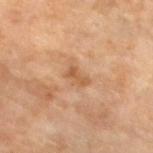Clinical impression:
This lesion was catalogued during total-body skin photography and was not selected for biopsy.
Image and clinical context:
On the left lower leg. A roughly 15 mm field-of-view crop from a total-body skin photograph. Approximately 2.5 mm at its widest. The tile uses cross-polarized illumination. A female patient approximately 70 years of age. Automated image analysis of the tile measured a lesion area of about 3.5 mm² and an outline eccentricity of about 0.85 (0 = round, 1 = elongated). The software also gave a mean CIELAB color near L≈55 a*≈21 b*≈36, a lesion–skin lightness drop of about 8, and a lesion-to-skin contrast of about 6.5 (normalized; higher = more distinct). The software also gave border irregularity of about 3.5 on a 0–10 scale, a color-variation rating of about 0.5/10, and peripheral color asymmetry of about 0.